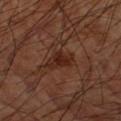The lesion was photographed on a routine skin check and not biopsied; there is no pathology result.
This image is a 15 mm lesion crop taken from a total-body photograph.
On the left upper arm.
A male patient, aged 58–62.